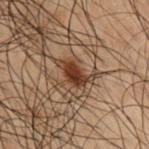No biopsy was performed on this lesion — it was imaged during a full skin examination and was not determined to be concerning. Longest diameter approximately 3 mm. A male patient, aged approximately 50. Captured under cross-polarized illumination. From the right upper arm. Cropped from a total-body skin-imaging series; the visible field is about 15 mm. The total-body-photography lesion software estimated a mean CIELAB color near L≈27 a*≈17 b*≈24, roughly 12 lightness units darker than nearby skin, and a lesion-to-skin contrast of about 12 (normalized; higher = more distinct). The software also gave a border-irregularity rating of about 2.5/10, internal color variation of about 3 on a 0–10 scale, and radial color variation of about 1.